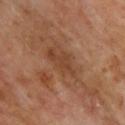Clinical impression:
No biopsy was performed on this lesion — it was imaged during a full skin examination and was not determined to be concerning.
Acquisition and patient details:
The tile uses cross-polarized illumination. On the upper back. A male subject, aged around 70. A 15 mm close-up extracted from a 3D total-body photography capture. Longest diameter approximately 9.5 mm.